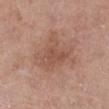• notes · no biopsy performed (imaged during a skin exam)
• patient · female, aged 38 to 42
• tile lighting · white-light
• imaging modality · ~15 mm crop, total-body skin-cancer survey
• anatomic site · the leg
• image-analysis metrics · a lesion color around L≈53 a*≈21 b*≈28 in CIELAB, roughly 7 lightness units darker than nearby skin, and a normalized lesion–skin contrast near 5.5; a border-irregularity rating of about 5/10; lesion-presence confidence of about 100/100